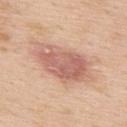Findings:
– notes: total-body-photography surveillance lesion; no biopsy
– subject: male, roughly 60 years of age
– imaging modality: ~15 mm tile from a whole-body skin photo
– diameter: ~7.5 mm (longest diameter)
– image-analysis metrics: an area of roughly 22 mm², an outline eccentricity of about 0.85 (0 = round, 1 = elongated), and a shape-asymmetry score of about 0.2 (0 = symmetric); a within-lesion color-variation index near 5/10 and radial color variation of about 1.5
– body site: the back
– lighting: white-light